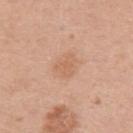Part of a total-body skin-imaging series; this lesion was reviewed on a skin check and was not flagged for biopsy. The recorded lesion diameter is about 3.5 mm. The lesion is located on the arm. The patient is a female roughly 40 years of age. Captured under white-light illumination. Cropped from a total-body skin-imaging series; the visible field is about 15 mm.